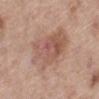Imaged during a routine full-body skin examination; the lesion was not biopsied and no histopathology is available. A roughly 15 mm field-of-view crop from a total-body skin photograph. Located on the left lower leg. Imaged with white-light lighting. Measured at roughly 7 mm in maximum diameter. A female subject in their mid-60s.